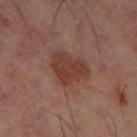Assessment:
The lesion was tiled from a total-body skin photograph and was not biopsied.
Context:
On the left thigh. Cropped from a total-body skin-imaging series; the visible field is about 15 mm. This is a cross-polarized tile.